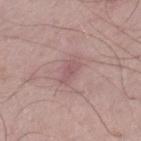The lesion was tiled from a total-body skin photograph and was not biopsied. A male patient approximately 50 years of age. About 2.5 mm across. The total-body-photography lesion software estimated an area of roughly 3 mm², a shape eccentricity near 0.85, and two-axis asymmetry of about 0.35. The lesion is on the left thigh. This image is a 15 mm lesion crop taken from a total-body photograph.